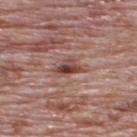Clinical impression:
The lesion was tiled from a total-body skin photograph and was not biopsied.
Image and clinical context:
The lesion is located on the upper back. This is a white-light tile. Automated tile analysis of the lesion measured a classifier nevus-likeness of about 35/100. A region of skin cropped from a whole-body photographic capture, roughly 15 mm wide. A male subject about 70 years old.